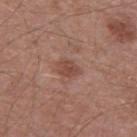workup = total-body-photography surveillance lesion; no biopsy
patient = male, aged around 55
imaging modality = ~15 mm crop, total-body skin-cancer survey
TBP lesion metrics = an average lesion color of about L≈47 a*≈22 b*≈26 (CIELAB), about 8 CIELAB-L* units darker than the surrounding skin, and a normalized border contrast of about 6.5
anatomic site = the back
illumination = white-light